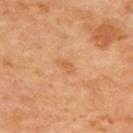Clinical impression: The lesion was tiled from a total-body skin photograph and was not biopsied.